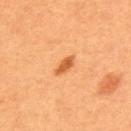{"biopsy_status": "not biopsied; imaged during a skin examination", "patient": {"sex": "female", "age_approx": 50}, "image": {"source": "total-body photography crop", "field_of_view_mm": 15}}